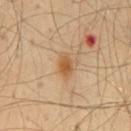location=the mid back | subject=male, about 45 years old | image=~15 mm tile from a whole-body skin photo | illumination=cross-polarized | automated metrics=a lesion color around L≈55 a*≈20 b*≈39 in CIELAB and a normalized border contrast of about 8.5; a border-irregularity rating of about 2/10, a within-lesion color-variation index near 2.5/10, and a peripheral color-asymmetry measure near 0.5; an automated nevus-likeness rating near 70 out of 100 and lesion-presence confidence of about 100/100 | lesion size=~3 mm (longest diameter).